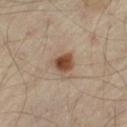No biopsy was performed on this lesion — it was imaged during a full skin examination and was not determined to be concerning. The recorded lesion diameter is about 3 mm. Captured under cross-polarized illumination. A 15 mm close-up tile from a total-body photography series done for melanoma screening. On the left thigh. The patient is approximately 55 years of age.